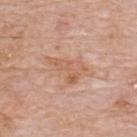notes=no biopsy performed (imaged during a skin exam).